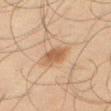Impression: Recorded during total-body skin imaging; not selected for excision or biopsy. Clinical summary: This is a cross-polarized tile. A male subject in their mid- to late 40s. Automated image analysis of the tile measured a lesion area of about 7 mm² and an eccentricity of roughly 0.8. The analysis additionally found roughly 9 lightness units darker than nearby skin and a lesion-to-skin contrast of about 7 (normalized; higher = more distinct). The software also gave a border-irregularity index near 2/10 and a peripheral color-asymmetry measure near 0.5. And it measured a nevus-likeness score of about 80/100 and lesion-presence confidence of about 100/100. The lesion is located on the leg. About 4 mm across. A lesion tile, about 15 mm wide, cut from a 3D total-body photograph.